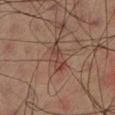Clinical summary:
A male patient in their 60s. This image is a 15 mm lesion crop taken from a total-body photograph. The total-body-photography lesion software estimated a border-irregularity index near 5/10, internal color variation of about 3 on a 0–10 scale, and peripheral color asymmetry of about 1. And it measured an automated nevus-likeness rating near 10 out of 100. The lesion's longest dimension is about 4.5 mm. This is a cross-polarized tile.